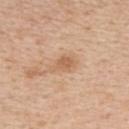The lesion was tiled from a total-body skin photograph and was not biopsied.